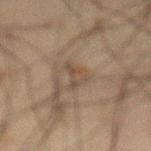diameter: about 3.5 mm; image: ~15 mm tile from a whole-body skin photo; subject: male, approximately 60 years of age; anatomic site: the abdomen; illumination: cross-polarized.